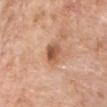Assessment:
This lesion was catalogued during total-body skin photography and was not selected for biopsy.
Context:
A 15 mm close-up tile from a total-body photography series done for melanoma screening. From the chest. Captured under white-light illumination. A male subject roughly 60 years of age.